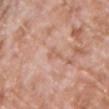follow-up: total-body-photography surveillance lesion; no biopsy
subject: male, roughly 70 years of age
anatomic site: the chest
image: total-body-photography crop, ~15 mm field of view
lighting: white-light illumination
size: about 2.5 mm